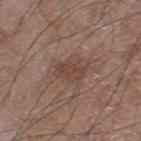Impression: Imaged during a routine full-body skin examination; the lesion was not biopsied and no histopathology is available. Context: The lesion's longest dimension is about 3 mm. A 15 mm close-up tile from a total-body photography series done for melanoma screening. Located on the right lower leg. Captured under white-light illumination. A male subject, aged 58 to 62.